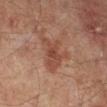Background: This is a cross-polarized tile. Measured at roughly 5 mm in maximum diameter. A close-up tile cropped from a whole-body skin photograph, about 15 mm across. The subject is a male aged around 50. Located on the left leg.